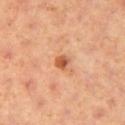Case summary:
* image — 15 mm crop, total-body photography
* diameter — about 2 mm
* subject — female, approximately 40 years of age
* automated lesion analysis — a mean CIELAB color near L≈57 a*≈28 b*≈39 and a lesion-to-skin contrast of about 8.5 (normalized; higher = more distinct); a lesion-detection confidence of about 100/100
* body site — the right thigh